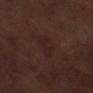Q: Is there a histopathology result?
A: no biopsy performed (imaged during a skin exam)
Q: Lesion size?
A: ≈3.5 mm
Q: Patient demographics?
A: male, aged 68–72
Q: Where on the body is the lesion?
A: the leg
Q: What lighting was used for the tile?
A: white-light illumination
Q: What kind of image is this?
A: total-body-photography crop, ~15 mm field of view
Q: Automated lesion metrics?
A: border irregularity of about 3 on a 0–10 scale, internal color variation of about 1 on a 0–10 scale, and peripheral color asymmetry of about 0.5; a classifier nevus-likeness of about 15/100 and a lesion-detection confidence of about 100/100